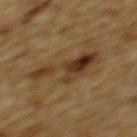Captured during whole-body skin photography for melanoma surveillance; the lesion was not biopsied. The subject is a male aged 83 or over. Measured at roughly 7.5 mm in maximum diameter. Imaged with cross-polarized lighting. The total-body-photography lesion software estimated an area of roughly 15 mm², an eccentricity of roughly 0.9, and a shape-asymmetry score of about 0.55 (0 = symmetric). From the upper back. A 15 mm close-up tile from a total-body photography series done for melanoma screening.